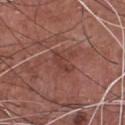Q: Was this lesion biopsied?
A: total-body-photography surveillance lesion; no biopsy
Q: Patient demographics?
A: male, in their mid- to late 70s
Q: What is the anatomic site?
A: the chest
Q: What kind of image is this?
A: ~15 mm crop, total-body skin-cancer survey
Q: Illumination type?
A: white-light illumination